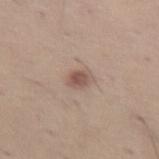A 15 mm close-up tile from a total-body photography series done for melanoma screening. A male patient approximately 45 years of age. The lesion is on the abdomen. An algorithmic analysis of the crop reported an eccentricity of roughly 0.65 and a symmetry-axis asymmetry near 0.25. The software also gave a border-irregularity index near 2/10, a color-variation rating of about 3.5/10, and radial color variation of about 1. And it measured a nevus-likeness score of about 60/100 and a detector confidence of about 100 out of 100 that the crop contains a lesion. Captured under white-light illumination. The lesion's longest dimension is about 2.5 mm.